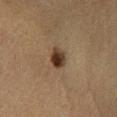Imaged during a routine full-body skin examination; the lesion was not biopsied and no histopathology is available.
The lesion-visualizer software estimated a border-irregularity rating of about 2/10 and a peripheral color-asymmetry measure near 2.
Located on the left lower leg.
A 15 mm crop from a total-body photograph taken for skin-cancer surveillance.
The patient is a male aged approximately 65.